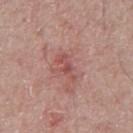The lesion was tiled from a total-body skin photograph and was not biopsied. Automated image analysis of the tile measured internal color variation of about 1.5 on a 0–10 scale and a peripheral color-asymmetry measure near 0.5. This is a white-light tile. Cropped from a whole-body photographic skin survey; the tile spans about 15 mm. On the front of the torso. Measured at roughly 3.5 mm in maximum diameter. The patient is a male roughly 65 years of age.